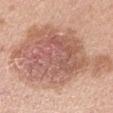<case>
<site>left upper arm</site>
<patient>
  <sex>male</sex>
  <age_approx>25</age_approx>
</patient>
<image>
  <source>total-body photography crop</source>
  <field_of_view_mm>15</field_of_view_mm>
</image>
<lesion_size>
  <long_diameter_mm_approx>12.0</long_diameter_mm_approx>
</lesion_size>
<lighting>white-light</lighting>
</case>